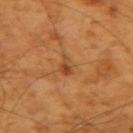{
  "biopsy_status": "not biopsied; imaged during a skin examination",
  "patient": {
    "sex": "male",
    "age_approx": 60
  },
  "image": {
    "source": "total-body photography crop",
    "field_of_view_mm": 15
  },
  "site": "left forearm"
}